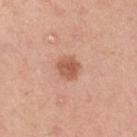The lesion was tiled from a total-body skin photograph and was not biopsied. From the right upper arm. Imaged with white-light lighting. About 3 mm across. A female subject, roughly 45 years of age. A close-up tile cropped from a whole-body skin photograph, about 15 mm across.